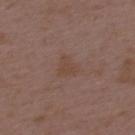Q: What did automated image analysis measure?
A: a lesion area of about 5 mm², a shape eccentricity near 0.55, and a symmetry-axis asymmetry near 0.4; a border-irregularity index near 3.5/10, internal color variation of about 1.5 on a 0–10 scale, and a peripheral color-asymmetry measure near 0.5
Q: Illumination type?
A: white-light
Q: What kind of image is this?
A: total-body-photography crop, ~15 mm field of view
Q: What is the lesion's diameter?
A: ~2.5 mm (longest diameter)
Q: What are the patient's age and sex?
A: male, aged 48–52
Q: Lesion location?
A: the upper back